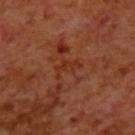Clinical impression: The lesion was tiled from a total-body skin photograph and was not biopsied. Acquisition and patient details: The patient is a male roughly 70 years of age. Automated image analysis of the tile measured an area of roughly 4 mm² and an outline eccentricity of about 0.9 (0 = round, 1 = elongated). And it measured roughly 5 lightness units darker than nearby skin and a normalized lesion–skin contrast near 5.5. And it measured a within-lesion color-variation index near 0/10 and a peripheral color-asymmetry measure near 0. It also reported a classifier nevus-likeness of about 0/100 and a detector confidence of about 90 out of 100 that the crop contains a lesion. A 15 mm close-up extracted from a 3D total-body photography capture. The lesion's longest dimension is about 3.5 mm. Located on the back.